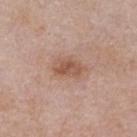This lesion was catalogued during total-body skin photography and was not selected for biopsy. The tile uses white-light illumination. Automated tile analysis of the lesion measured a footprint of about 7.5 mm², a shape eccentricity near 0.8, and two-axis asymmetry of about 0.15. The software also gave roughly 9 lightness units darker than nearby skin and a normalized border contrast of about 7. The analysis additionally found a border-irregularity index near 1.5/10, a color-variation rating of about 4/10, and peripheral color asymmetry of about 1.5. It also reported a nevus-likeness score of about 50/100 and lesion-presence confidence of about 100/100. A male subject, aged 53–57. A 15 mm close-up tile from a total-body photography series done for melanoma screening. Located on the chest.